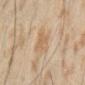Notes:
– biopsy status — imaged on a skin check; not biopsied
– illumination — cross-polarized illumination
– lesion size — ≈4.5 mm
– subject — male, in their mid- to late 40s
– imaging modality — ~15 mm crop, total-body skin-cancer survey
– automated lesion analysis — an outline eccentricity of about 0.8 (0 = round, 1 = elongated) and two-axis asymmetry of about 0.35; an automated nevus-likeness rating near 0 out of 100 and a detector confidence of about 100 out of 100 that the crop contains a lesion
– site — the arm